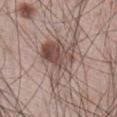{
  "biopsy_status": "not biopsied; imaged during a skin examination",
  "automated_metrics": {
    "nevus_likeness_0_100": 95,
    "lesion_detection_confidence_0_100": 100
  },
  "lesion_size": {
    "long_diameter_mm_approx": 7.0
  },
  "image": {
    "source": "total-body photography crop",
    "field_of_view_mm": 15
  },
  "site": "abdomen",
  "lighting": "white-light",
  "patient": {
    "sex": "male",
    "age_approx": 65
  }
}